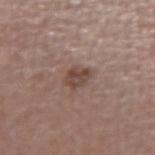Recorded during total-body skin imaging; not selected for excision or biopsy. The lesion is located on the left forearm. The total-body-photography lesion software estimated a nevus-likeness score of about 30/100 and a detector confidence of about 100 out of 100 that the crop contains a lesion. Captured under white-light illumination. Measured at roughly 2.5 mm in maximum diameter. A 15 mm close-up extracted from a 3D total-body photography capture. A female patient about 50 years old.